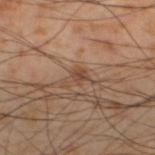<record>
<biopsy_status>not biopsied; imaged during a skin examination</biopsy_status>
<lighting>cross-polarized</lighting>
<image>
  <source>total-body photography crop</source>
  <field_of_view_mm>15</field_of_view_mm>
</image>
<site>left lower leg</site>
<lesion_size>
  <long_diameter_mm_approx>3.0</long_diameter_mm_approx>
</lesion_size>
<automated_metrics>
  <area_mm2_approx>4.0</area_mm2_approx>
  <eccentricity>0.75</eccentricity>
  <shape_asymmetry>0.55</shape_asymmetry>
  <cielab_L>46</cielab_L>
  <cielab_a>18</cielab_a>
  <cielab_b>29</cielab_b>
  <vs_skin_darker_L>8.0</vs_skin_darker_L>
  <vs_skin_contrast_norm>6.0</vs_skin_contrast_norm>
  <border_irregularity_0_10>7.5</border_irregularity_0_10>
  <color_variation_0_10>2.0</color_variation_0_10>
  <peripheral_color_asymmetry>0.5</peripheral_color_asymmetry>
</automated_metrics>
<patient>
  <sex>male</sex>
  <age_approx>55</age_approx>
</patient>
</record>